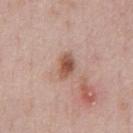Case summary:
* workup · no biopsy performed (imaged during a skin exam)
* subject · male, aged around 50
* lesion diameter · ≈3 mm
* lighting · white-light illumination
* TBP lesion metrics · a lesion area of about 5.5 mm² and two-axis asymmetry of about 0.2; an average lesion color of about L≈54 a*≈21 b*≈28 (CIELAB), roughly 12 lightness units darker than nearby skin, and a normalized border contrast of about 9; a border-irregularity index near 2/10 and peripheral color asymmetry of about 1
* location · the chest
* image · ~15 mm crop, total-body skin-cancer survey